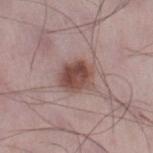Case summary:
- workup — imaged on a skin check; not biopsied
- lighting — white-light illumination
- image — total-body-photography crop, ~15 mm field of view
- subject — male, about 50 years old
- body site — the right lower leg
- diameter — ≈4 mm